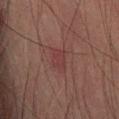Q: Is there a histopathology result?
A: total-body-photography surveillance lesion; no biopsy
Q: What did automated image analysis measure?
A: a footprint of about 4.5 mm² and a shape eccentricity near 0.85; a mean CIELAB color near L≈27 a*≈18 b*≈16
Q: Where on the body is the lesion?
A: the left leg
Q: How was this image acquired?
A: 15 mm crop, total-body photography
Q: What are the patient's age and sex?
A: male, roughly 60 years of age